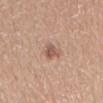The lesion was photographed on a routine skin check and not biopsied; there is no pathology result.
Imaged with white-light lighting.
An algorithmic analysis of the crop reported a lesion area of about 4 mm², a shape eccentricity near 0.55, and a symmetry-axis asymmetry near 0.4. It also reported a within-lesion color-variation index near 3/10 and radial color variation of about 1.
Longest diameter approximately 2.5 mm.
A close-up tile cropped from a whole-body skin photograph, about 15 mm across.
From the abdomen.
A male subject, approximately 20 years of age.